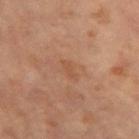biopsy status: no biopsy performed (imaged during a skin exam)
imaging modality: ~15 mm crop, total-body skin-cancer survey
TBP lesion metrics: a lesion area of about 2.5 mm², an eccentricity of roughly 0.9, and two-axis asymmetry of about 0.3; a normalized border contrast of about 4.5
size: ≈2.5 mm
illumination: cross-polarized
body site: the left thigh
patient: female, aged 63–67